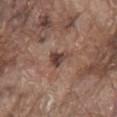lesion size: about 2.5 mm
site: the mid back
automated lesion analysis: a mean CIELAB color near L≈41 a*≈19 b*≈23 and a normalized lesion–skin contrast near 9.5; a lesion-detection confidence of about 100/100
image source: ~15 mm tile from a whole-body skin photo
tile lighting: white-light illumination
subject: male, roughly 80 years of age